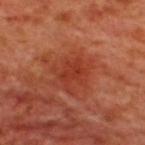The lesion was photographed on a routine skin check and not biopsied; there is no pathology result. The patient is a male in their 50s. The lesion-visualizer software estimated a lesion area of about 5.5 mm², a shape eccentricity near 0.65, and a symmetry-axis asymmetry near 0.4. It also reported a lesion color around L≈39 a*≈34 b*≈35 in CIELAB and a lesion–skin lightness drop of about 7. The software also gave border irregularity of about 5 on a 0–10 scale and a within-lesion color-variation index near 2/10. The analysis additionally found an automated nevus-likeness rating near 50 out of 100 and a detector confidence of about 95 out of 100 that the crop contains a lesion. A 15 mm close-up tile from a total-body photography series done for melanoma screening. The lesion is on the upper back. Imaged with cross-polarized lighting.